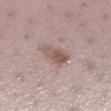Recorded during total-body skin imaging; not selected for excision or biopsy.